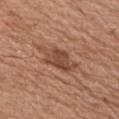Impression:
The lesion was photographed on a routine skin check and not biopsied; there is no pathology result.
Acquisition and patient details:
This is a white-light tile. The subject is a male in their mid-60s. A 15 mm close-up tile from a total-body photography series done for melanoma screening. From the chest.